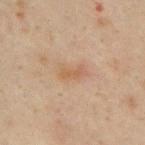Background: On the upper back. A male subject, aged approximately 50. A region of skin cropped from a whole-body photographic capture, roughly 15 mm wide.